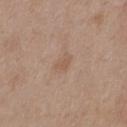{
  "biopsy_status": "not biopsied; imaged during a skin examination",
  "site": "mid back",
  "lesion_size": {
    "long_diameter_mm_approx": 2.5
  },
  "image": {
    "source": "total-body photography crop",
    "field_of_view_mm": 15
  },
  "patient": {
    "sex": "female",
    "age_approx": 45
  }
}